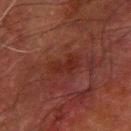site: the left forearm
patient: male, aged approximately 80
acquisition: ~15 mm crop, total-body skin-cancer survey
size: ≈3 mm
image-analysis metrics: a footprint of about 5.5 mm², an outline eccentricity of about 0.85 (0 = round, 1 = elongated), and a symmetry-axis asymmetry near 0.3; border irregularity of about 4 on a 0–10 scale; an automated nevus-likeness rating near 5 out of 100